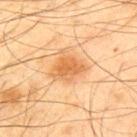Impression:
The lesion was photographed on a routine skin check and not biopsied; there is no pathology result.
Background:
This is a cross-polarized tile. The lesion is on the upper back. A 15 mm crop from a total-body photograph taken for skin-cancer surveillance. The lesion's longest dimension is about 4 mm. A male patient, in their mid- to late 40s.